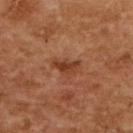Recorded during total-body skin imaging; not selected for excision or biopsy. Imaged with cross-polarized lighting. The subject is a female about 60 years old. Approximately 3.5 mm at its widest. Automated image analysis of the tile measured a lesion area of about 5.5 mm² and a shape-asymmetry score of about 0.4 (0 = symmetric). The analysis additionally found a mean CIELAB color near L≈35 a*≈22 b*≈29 and a lesion-to-skin contrast of about 7 (normalized; higher = more distinct). It also reported a border-irregularity rating of about 4/10 and radial color variation of about 1. It also reported a detector confidence of about 100 out of 100 that the crop contains a lesion. A close-up tile cropped from a whole-body skin photograph, about 15 mm across. From the upper back.